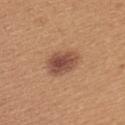<tbp_lesion>
<biopsy_status>not biopsied; imaged during a skin examination</biopsy_status>
<lighting>white-light</lighting>
<patient>
  <sex>female</sex>
  <age_approx>25</age_approx>
</patient>
<image>
  <source>total-body photography crop</source>
  <field_of_view_mm>15</field_of_view_mm>
</image>
<site>arm</site>
<lesion_size>
  <long_diameter_mm_approx>4.0</long_diameter_mm_approx>
</lesion_size>
</tbp_lesion>